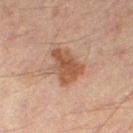{
  "biopsy_status": "not biopsied; imaged during a skin examination",
  "site": "left leg",
  "image": {
    "source": "total-body photography crop",
    "field_of_view_mm": 15
  },
  "patient": {
    "sex": "male",
    "age_approx": 60
  },
  "lesion_size": {
    "long_diameter_mm_approx": 4.5
  }
}